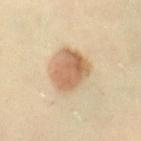follow-up — imaged on a skin check; not biopsied | patient — female, aged 38–42 | acquisition — total-body-photography crop, ~15 mm field of view | site — the right thigh | tile lighting — cross-polarized.